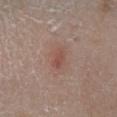workup: catalogued during a skin exam; not biopsied | TBP lesion metrics: a footprint of about 3.5 mm², an outline eccentricity of about 0.8 (0 = round, 1 = elongated), and two-axis asymmetry of about 0.25; a lesion-detection confidence of about 100/100 | anatomic site: the right lower leg | subject: female, roughly 70 years of age | image: ~15 mm tile from a whole-body skin photo | lesion diameter: about 2.5 mm.